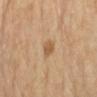Case summary:
• biopsy status — catalogued during a skin exam; not biopsied
• diameter — ≈2 mm
• patient — female, in their mid- to late 60s
• image — ~15 mm crop, total-body skin-cancer survey
• body site — the left forearm
• illumination — cross-polarized